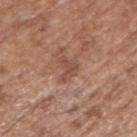Q: Was a biopsy performed?
A: no biopsy performed (imaged during a skin exam)
Q: What did automated image analysis measure?
A: a mean CIELAB color near L≈49 a*≈21 b*≈27, a lesion–skin lightness drop of about 8, and a normalized lesion–skin contrast near 6; a border-irregularity index near 6.5/10, a color-variation rating of about 1.5/10, and radial color variation of about 0.5
Q: What is the anatomic site?
A: the right upper arm
Q: What is the imaging modality?
A: 15 mm crop, total-body photography
Q: Patient demographics?
A: male, aged approximately 75